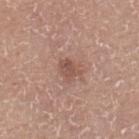Impression: Part of a total-body skin-imaging series; this lesion was reviewed on a skin check and was not flagged for biopsy. Clinical summary: Captured under white-light illumination. From the right lower leg. About 2.5 mm across. Automated tile analysis of the lesion measured a classifier nevus-likeness of about 30/100 and a lesion-detection confidence of about 100/100. A female subject in their mid- to late 70s. A 15 mm crop from a total-body photograph taken for skin-cancer surveillance.